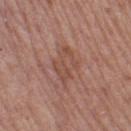This lesion was catalogued during total-body skin photography and was not selected for biopsy. Captured under white-light illumination. Cropped from a whole-body photographic skin survey; the tile spans about 15 mm. An algorithmic analysis of the crop reported a lesion-detection confidence of about 95/100. On the left thigh. The subject is a female about 60 years old.